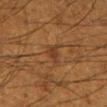Q: Was a biopsy performed?
A: no biopsy performed (imaged during a skin exam)
Q: What kind of image is this?
A: ~15 mm crop, total-body skin-cancer survey
Q: What are the patient's age and sex?
A: male, approximately 60 years of age
Q: Automated lesion metrics?
A: an area of roughly 3.5 mm², an outline eccentricity of about 0.7 (0 = round, 1 = elongated), and a symmetry-axis asymmetry near 0.4
Q: How large is the lesion?
A: about 2.5 mm
Q: How was the tile lit?
A: cross-polarized illumination
Q: What is the anatomic site?
A: the left lower leg